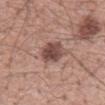Impression:
No biopsy was performed on this lesion — it was imaged during a full skin examination and was not determined to be concerning.